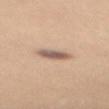No biopsy was performed on this lesion — it was imaged during a full skin examination and was not determined to be concerning. Automated image analysis of the tile measured an average lesion color of about L≈59 a*≈17 b*≈25 (CIELAB), a lesion–skin lightness drop of about 14, and a lesion-to-skin contrast of about 9 (normalized; higher = more distinct). The analysis additionally found a classifier nevus-likeness of about 90/100 and lesion-presence confidence of about 100/100. Located on the mid back. A 15 mm close-up extracted from a 3D total-body photography capture. A female subject aged around 30. The recorded lesion diameter is about 3.5 mm.